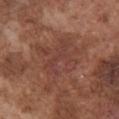Captured during whole-body skin photography for melanoma surveillance; the lesion was not biopsied. The lesion is on the chest. A 15 mm close-up tile from a total-body photography series done for melanoma screening. A male subject in their mid- to late 70s.